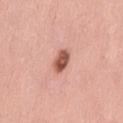Q: Was this lesion biopsied?
A: no biopsy performed (imaged during a skin exam)
Q: Where on the body is the lesion?
A: the mid back
Q: What kind of image is this?
A: total-body-photography crop, ~15 mm field of view
Q: What are the patient's age and sex?
A: male, aged approximately 60